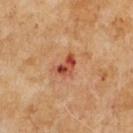Part of a total-body skin-imaging series; this lesion was reviewed on a skin check and was not flagged for biopsy.
The patient is a male approximately 65 years of age.
Cropped from a total-body skin-imaging series; the visible field is about 15 mm.
About 3 mm across.
The lesion-visualizer software estimated a footprint of about 4.5 mm², an outline eccentricity of about 0.75 (0 = round, 1 = elongated), and a symmetry-axis asymmetry near 0.45. It also reported a mean CIELAB color near L≈48 a*≈29 b*≈34, about 12 CIELAB-L* units darker than the surrounding skin, and a normalized lesion–skin contrast near 8.5.
This is a cross-polarized tile.